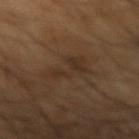  biopsy_status: not biopsied; imaged during a skin examination
  image:
    source: total-body photography crop
    field_of_view_mm: 15
  patient:
    sex: male
    age_approx: 65
  site: right forearm